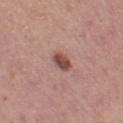workup = no biopsy performed (imaged during a skin exam)
size = ≈2.5 mm
illumination = white-light
image = ~15 mm crop, total-body skin-cancer survey
subject = female, aged approximately 30
image-analysis metrics = an area of roughly 4 mm² and an eccentricity of roughly 0.75
anatomic site = the leg